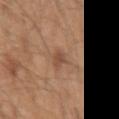Background: A region of skin cropped from a whole-body photographic capture, roughly 15 mm wide. This is a white-light tile. Located on the left upper arm. The lesion's longest dimension is about 2.5 mm. The patient is a male aged approximately 50. The total-body-photography lesion software estimated an eccentricity of roughly 0.8 and a shape-asymmetry score of about 0.3 (0 = symmetric). The software also gave a border-irregularity rating of about 3/10 and peripheral color asymmetry of about 0.5.